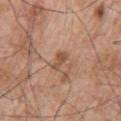Case summary:
- workup — no biopsy performed (imaged during a skin exam)
- anatomic site — the left arm
- subject — male, about 70 years old
- image — ~15 mm crop, total-body skin-cancer survey